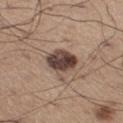  biopsy_status: not biopsied; imaged during a skin examination
  patient:
    sex: male
    age_approx: 65
  automated_metrics:
    cielab_L: 42
    cielab_a: 16
    cielab_b: 22
    vs_skin_darker_L: 17.0
    border_irregularity_0_10: 1.5
    color_variation_0_10: 6.5
    peripheral_color_asymmetry: 2.5
    lesion_detection_confidence_0_100: 100
  site: left thigh
  lesion_size:
    long_diameter_mm_approx: 3.5
  image:
    source: total-body photography crop
    field_of_view_mm: 15